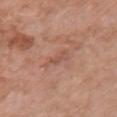Located on the front of the torso. Captured under white-light illumination. A female subject approximately 50 years of age. Automated tile analysis of the lesion measured an area of roughly 4 mm² and a shape eccentricity near 0.95. The software also gave a mean CIELAB color near L≈53 a*≈23 b*≈28 and a normalized border contrast of about 4.5. The software also gave a border-irregularity index near 5.5/10, a within-lesion color-variation index near 0.5/10, and radial color variation of about 0. The lesion's longest dimension is about 3.5 mm. A 15 mm crop from a total-body photograph taken for skin-cancer surveillance.